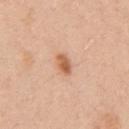Imaged during a routine full-body skin examination; the lesion was not biopsied and no histopathology is available.
A male subject aged around 50.
The lesion's longest dimension is about 3 mm.
Imaged with white-light lighting.
Cropped from a whole-body photographic skin survey; the tile spans about 15 mm.
The lesion is on the chest.